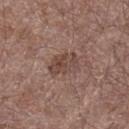| field | value |
|---|---|
| workup | total-body-photography surveillance lesion; no biopsy |
| lesion diameter | about 3.5 mm |
| location | the leg |
| imaging modality | ~15 mm crop, total-body skin-cancer survey |
| patient | male, approximately 70 years of age |